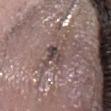– diameter · about 2.5 mm
– patient · female, aged approximately 50
– automated lesion analysis · an area of roughly 4.5 mm², an eccentricity of roughly 0.7, and a symmetry-axis asymmetry near 0.35; a mean CIELAB color near L≈43 a*≈13 b*≈16, roughly 9 lightness units darker than nearby skin, and a normalized lesion–skin contrast near 7.5; lesion-presence confidence of about 55/100
– illumination · white-light illumination
– image · ~15 mm tile from a whole-body skin photo
– site · the head or neck